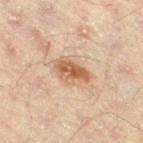biopsy_status: not biopsied; imaged during a skin examination
lighting: cross-polarized
lesion_size:
  long_diameter_mm_approx: 4.0
image:
  source: total-body photography crop
  field_of_view_mm: 15
patient:
  sex: male
  age_approx: 45
automated_metrics:
  area_mm2_approx: 7.5
  eccentricity: 0.8
  shape_asymmetry: 0.25
site: right thigh